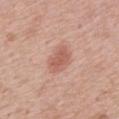follow-up — catalogued during a skin exam; not biopsied | patient — male, about 60 years old | image source — total-body-photography crop, ~15 mm field of view | illumination — white-light | anatomic site — the mid back.